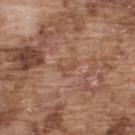* patient: male, aged 73 to 77
* size: ~2.5 mm (longest diameter)
* illumination: white-light illumination
* image source: ~15 mm crop, total-body skin-cancer survey
* anatomic site: the upper back